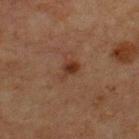Q: Is there a histopathology result?
A: imaged on a skin check; not biopsied
Q: Patient demographics?
A: male, in their mid- to late 60s
Q: What is the anatomic site?
A: the chest
Q: Illumination type?
A: cross-polarized illumination
Q: How was this image acquired?
A: 15 mm crop, total-body photography